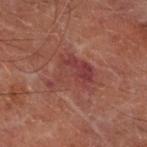Impression:
Captured during whole-body skin photography for melanoma surveillance; the lesion was not biopsied.
Acquisition and patient details:
A 15 mm close-up tile from a total-body photography series done for melanoma screening. A male patient, aged 68–72. The lesion's longest dimension is about 5.5 mm. Captured under cross-polarized illumination. The total-body-photography lesion software estimated an area of roughly 13 mm², a shape eccentricity near 0.7, and two-axis asymmetry of about 0.4. And it measured a lesion color around L≈30 a*≈21 b*≈19 in CIELAB, a lesion–skin lightness drop of about 6, and a normalized border contrast of about 6. The analysis additionally found lesion-presence confidence of about 100/100. On the right forearm.